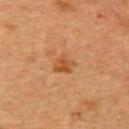{"lighting": "cross-polarized", "lesion_size": {"long_diameter_mm_approx": 3.0}, "image": {"source": "total-body photography crop", "field_of_view_mm": 15}, "site": "back", "patient": {"sex": "female", "age_approx": 55}}